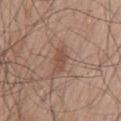Imaged during a routine full-body skin examination; the lesion was not biopsied and no histopathology is available.
A region of skin cropped from a whole-body photographic capture, roughly 15 mm wide.
Captured under white-light illumination.
The patient is a male about 70 years old.
An algorithmic analysis of the crop reported an area of roughly 4.5 mm² and a shape eccentricity near 0.85. The analysis additionally found a color-variation rating of about 1.5/10 and radial color variation of about 0.5. The software also gave a classifier nevus-likeness of about 5/100 and a detector confidence of about 100 out of 100 that the crop contains a lesion.
From the back.